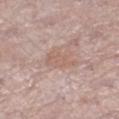No biopsy was performed on this lesion — it was imaged during a full skin examination and was not determined to be concerning. This image is a 15 mm lesion crop taken from a total-body photograph. The lesion is located on the right lower leg. A female subject in their 60s.